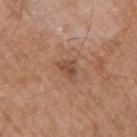- notes — no biopsy performed (imaged during a skin exam)
- illumination — white-light
- location — the right upper arm
- subject — male, aged 63–67
- image — ~15 mm tile from a whole-body skin photo